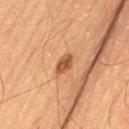Clinical impression: The lesion was photographed on a routine skin check and not biopsied; there is no pathology result. Background: This is a cross-polarized tile. The lesion is on the left thigh. Longest diameter approximately 3 mm. Automated tile analysis of the lesion measured a footprint of about 3.5 mm² and a shape-asymmetry score of about 0.25 (0 = symmetric). The software also gave an average lesion color of about L≈44 a*≈21 b*≈33 (CIELAB) and a normalized lesion–skin contrast near 8.5. And it measured border irregularity of about 2.5 on a 0–10 scale, internal color variation of about 2.5 on a 0–10 scale, and radial color variation of about 1. The analysis additionally found a classifier nevus-likeness of about 80/100. A male subject aged 63–67. Cropped from a total-body skin-imaging series; the visible field is about 15 mm.